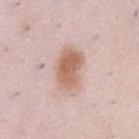Acquisition and patient details: Cropped from a total-body skin-imaging series; the visible field is about 15 mm. Imaged with white-light lighting. The lesion is located on the front of the torso. The patient is a male aged around 50. The lesion's longest dimension is about 5 mm.